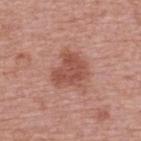Part of a total-body skin-imaging series; this lesion was reviewed on a skin check and was not flagged for biopsy.
Automated image analysis of the tile measured a lesion area of about 12 mm², a shape eccentricity near 0.4, and a shape-asymmetry score of about 0.35 (0 = symmetric). The software also gave an average lesion color of about L≈51 a*≈26 b*≈28 (CIELAB), roughly 10 lightness units darker than nearby skin, and a lesion-to-skin contrast of about 7.5 (normalized; higher = more distinct). The analysis additionally found an automated nevus-likeness rating near 40 out of 100 and a detector confidence of about 100 out of 100 that the crop contains a lesion.
On the upper back.
About 4 mm across.
A female subject aged 58 to 62.
A roughly 15 mm field-of-view crop from a total-body skin photograph.
This is a white-light tile.